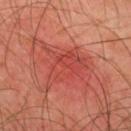Captured under cross-polarized illumination.
On the back.
Measured at roughly 5.5 mm in maximum diameter.
The patient is a male aged 43 to 47.
A roughly 15 mm field-of-view crop from a total-body skin photograph.